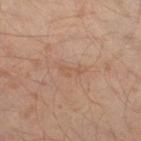Recorded during total-body skin imaging; not selected for excision or biopsy. On the left leg. A 15 mm crop from a total-body photograph taken for skin-cancer surveillance. A male subject, aged 28 to 32.